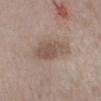Q: Was this lesion biopsied?
A: catalogued during a skin exam; not biopsied
Q: What is the lesion's diameter?
A: about 5.5 mm
Q: Illumination type?
A: white-light
Q: What did automated image analysis measure?
A: a border-irregularity rating of about 2/10, a within-lesion color-variation index near 3.5/10, and a peripheral color-asymmetry measure near 1
Q: Where on the body is the lesion?
A: the left lower leg
Q: Patient demographics?
A: female, about 55 years old
Q: What kind of image is this?
A: total-body-photography crop, ~15 mm field of view